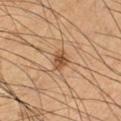biopsy_status: not biopsied; imaged during a skin examination
patient:
  sex: male
  age_approx: 60
lesion_size:
  long_diameter_mm_approx: 2.5
site: right lower leg
image:
  source: total-body photography crop
  field_of_view_mm: 15
automated_metrics:
  vs_skin_contrast_norm: 8.0
  border_irregularity_0_10: 1.5
  color_variation_0_10: 3.0
  peripheral_color_asymmetry: 1.0